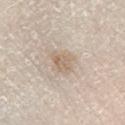Image and clinical context: The lesion's longest dimension is about 3 mm. On the right lower leg. Automated tile analysis of the lesion measured a lesion color around L≈65 a*≈12 b*≈28 in CIELAB and a lesion–skin lightness drop of about 8. A male subject, aged 58 to 62. Captured under white-light illumination. Cropped from a whole-body photographic skin survey; the tile spans about 15 mm.